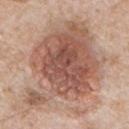Assessment:
This lesion was catalogued during total-body skin photography and was not selected for biopsy.
Clinical summary:
Captured under white-light illumination. From the chest. Longest diameter approximately 12 mm. A male subject about 65 years old. A region of skin cropped from a whole-body photographic capture, roughly 15 mm wide.